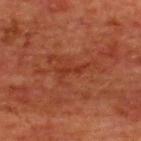Captured during whole-body skin photography for melanoma surveillance; the lesion was not biopsied. The patient is a male in their mid-60s. Automated tile analysis of the lesion measured a lesion–skin lightness drop of about 6 and a lesion-to-skin contrast of about 5.5 (normalized; higher = more distinct). And it measured a classifier nevus-likeness of about 0/100 and a detector confidence of about 80 out of 100 that the crop contains a lesion. From the upper back. Imaged with cross-polarized lighting. A 15 mm close-up tile from a total-body photography series done for melanoma screening. The recorded lesion diameter is about 3 mm.